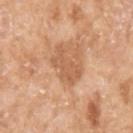| field | value |
|---|---|
| workup | catalogued during a skin exam; not biopsied |
| imaging modality | 15 mm crop, total-body photography |
| lesion diameter | ≈4 mm |
| subject | female, approximately 75 years of age |
| lighting | white-light illumination |
| site | the arm |